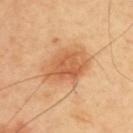Q: Is there a histopathology result?
A: total-body-photography surveillance lesion; no biopsy
Q: What is the imaging modality?
A: ~15 mm crop, total-body skin-cancer survey
Q: Patient demographics?
A: male, aged 63 to 67
Q: Lesion location?
A: the upper back
Q: What is the lesion's diameter?
A: ≈6 mm
Q: Automated lesion metrics?
A: a border-irregularity rating of about 2.5/10, a within-lesion color-variation index near 4.5/10, and a peripheral color-asymmetry measure near 1.5